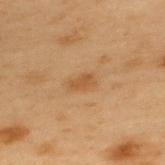Impression:
The lesion was photographed on a routine skin check and not biopsied; there is no pathology result.
Acquisition and patient details:
The lesion's longest dimension is about 3 mm. On the upper back. A 15 mm close-up tile from a total-body photography series done for melanoma screening. A male subject, aged 53–57. Captured under cross-polarized illumination.